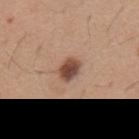The lesion was photographed on a routine skin check and not biopsied; there is no pathology result.
A 15 mm crop from a total-body photograph taken for skin-cancer surveillance.
The recorded lesion diameter is about 3 mm.
A male subject aged approximately 65.
The lesion is located on the mid back.